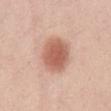Captured during whole-body skin photography for melanoma surveillance; the lesion was not biopsied. On the front of the torso. Captured under white-light illumination. A female subject about 35 years old. A roughly 15 mm field-of-view crop from a total-body skin photograph. Measured at roughly 4.5 mm in maximum diameter.